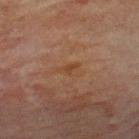Part of a total-body skin-imaging series; this lesion was reviewed on a skin check and was not flagged for biopsy. A 15 mm crop from a total-body photograph taken for skin-cancer surveillance. A male subject roughly 70 years of age. This is a cross-polarized tile. The lesion is located on the upper back.